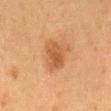Findings:
- notes · no biopsy performed (imaged during a skin exam)
- location · the mid back
- image · ~15 mm tile from a whole-body skin photo
- patient · female, approximately 50 years of age
- lesion diameter · about 4 mm
- lighting · cross-polarized
- TBP lesion metrics · a border-irregularity rating of about 3.5/10, a color-variation rating of about 3/10, and a peripheral color-asymmetry measure near 1; a classifier nevus-likeness of about 75/100 and a detector confidence of about 100 out of 100 that the crop contains a lesion